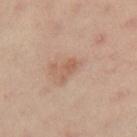Part of a total-body skin-imaging series; this lesion was reviewed on a skin check and was not flagged for biopsy. The subject is a female in their mid-50s. Imaged with cross-polarized lighting. This image is a 15 mm lesion crop taken from a total-body photograph. Automated tile analysis of the lesion measured a nevus-likeness score of about 5/100 and a detector confidence of about 100 out of 100 that the crop contains a lesion. On the right thigh.